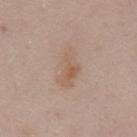The lesion was tiled from a total-body skin photograph and was not biopsied.
A region of skin cropped from a whole-body photographic capture, roughly 15 mm wide.
A male subject, roughly 55 years of age.
This is a white-light tile.
From the front of the torso.